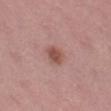The lesion was tiled from a total-body skin photograph and was not biopsied. A lesion tile, about 15 mm wide, cut from a 3D total-body photograph. An algorithmic analysis of the crop reported an average lesion color of about L≈51 a*≈23 b*≈25 (CIELAB), roughly 10 lightness units darker than nearby skin, and a normalized lesion–skin contrast near 8. The analysis additionally found a classifier nevus-likeness of about 85/100. Located on the left thigh. A female subject aged 43 to 47. Imaged with white-light lighting.